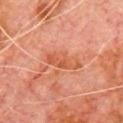Findings:
• workup — total-body-photography surveillance lesion; no biopsy
• lighting — cross-polarized illumination
• patient — male, aged approximately 80
• image — 15 mm crop, total-body photography
• body site — the chest